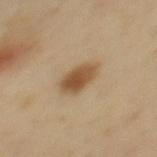No biopsy was performed on this lesion — it was imaged during a full skin examination and was not determined to be concerning. On the mid back. Cropped from a whole-body photographic skin survey; the tile spans about 15 mm. A female subject in their 40s. Automated tile analysis of the lesion measured a footprint of about 8 mm², an eccentricity of roughly 0.8, and a symmetry-axis asymmetry near 0.15. The analysis additionally found an average lesion color of about L≈52 a*≈17 b*≈35 (CIELAB), roughly 12 lightness units darker than nearby skin, and a normalized lesion–skin contrast near 9. Measured at roughly 4 mm in maximum diameter.